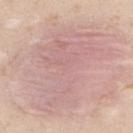<case>
  <biopsy_status>not biopsied; imaged during a skin examination</biopsy_status>
  <patient>
    <sex>male</sex>
    <age_approx>25</age_approx>
  </patient>
  <lesion_size>
    <long_diameter_mm_approx>12.0</long_diameter_mm_approx>
  </lesion_size>
  <lighting>white-light</lighting>
  <image>
    <source>total-body photography crop</source>
    <field_of_view_mm>15</field_of_view_mm>
  </image>
  <automated_metrics>
    <area_mm2_approx>60.0</area_mm2_approx>
    <eccentricity>0.75</eccentricity>
    <border_irregularity_0_10>7.5</border_irregularity_0_10>
    <color_variation_0_10>4.5</color_variation_0_10>
    <peripheral_color_asymmetry>1.5</peripheral_color_asymmetry>
    <nevus_likeness_0_100>0</nevus_likeness_0_100>
    <lesion_detection_confidence_0_100>60</lesion_detection_confidence_0_100>
  </automated_metrics>
  <site>upper back</site>
</case>